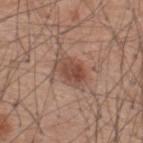| feature | finding |
|---|---|
| notes | total-body-photography surveillance lesion; no biopsy |
| size | about 4 mm |
| automated lesion analysis | a shape eccentricity near 0.8 and two-axis asymmetry of about 0.2; lesion-presence confidence of about 100/100 |
| illumination | white-light |
| image source | 15 mm crop, total-body photography |
| site | the upper back |
| patient | male, aged approximately 60 |